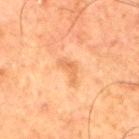Findings:
- workup · imaged on a skin check; not biopsied
- size · ~3.5 mm (longest diameter)
- imaging modality · total-body-photography crop, ~15 mm field of view
- subject · male, in their 80s
- tile lighting · cross-polarized illumination
- body site · the right thigh
- TBP lesion metrics · a footprint of about 4.5 mm² and a shape-asymmetry score of about 0.55 (0 = symmetric); a nevus-likeness score of about 0/100 and a lesion-detection confidence of about 100/100